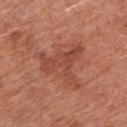biopsy status: no biopsy performed (imaged during a skin exam) | subject: female, aged around 65 | site: the left arm | imaging modality: ~15 mm tile from a whole-body skin photo | automated metrics: a lesion color around L≈47 a*≈28 b*≈31 in CIELAB, a lesion–skin lightness drop of about 7, and a lesion-to-skin contrast of about 5.5 (normalized; higher = more distinct); a classifier nevus-likeness of about 0/100 and a detector confidence of about 100 out of 100 that the crop contains a lesion.